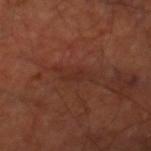Findings:
- notes · imaged on a skin check; not biopsied
- patient · about 65 years old
- illumination · cross-polarized illumination
- diameter · ~4 mm (longest diameter)
- location · the right thigh
- automated lesion analysis · a lesion color around L≈29 a*≈23 b*≈25 in CIELAB, a lesion–skin lightness drop of about 5, and a normalized border contrast of about 5; border irregularity of about 6.5 on a 0–10 scale and peripheral color asymmetry of about 0.5; a classifier nevus-likeness of about 0/100 and a detector confidence of about 90 out of 100 that the crop contains a lesion
- acquisition · total-body-photography crop, ~15 mm field of view